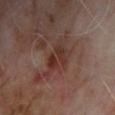follow-up — imaged on a skin check; not biopsied
anatomic site — the chest
lighting — cross-polarized illumination
acquisition — ~15 mm crop, total-body skin-cancer survey
patient — male, about 65 years old
automated metrics — a lesion area of about 10 mm², an eccentricity of roughly 0.85, and a symmetry-axis asymmetry near 0.45; border irregularity of about 5.5 on a 0–10 scale, a color-variation rating of about 5/10, and radial color variation of about 1.5; a nevus-likeness score of about 0/100 and a detector confidence of about 95 out of 100 that the crop contains a lesion